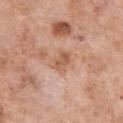Assessment:
Recorded during total-body skin imaging; not selected for excision or biopsy.
Context:
The recorded lesion diameter is about 3.5 mm. A 15 mm crop from a total-body photograph taken for skin-cancer surveillance. The lesion is located on the arm. A female subject, aged approximately 70. The tile uses white-light illumination. Automated tile analysis of the lesion measured a mean CIELAB color near L≈59 a*≈22 b*≈32 and a normalized border contrast of about 6.5. And it measured a color-variation rating of about 4/10 and a peripheral color-asymmetry measure near 1.5. The software also gave a nevus-likeness score of about 0/100 and a lesion-detection confidence of about 100/100.